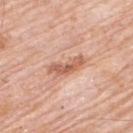This lesion was catalogued during total-body skin photography and was not selected for biopsy.
Longest diameter approximately 4.5 mm.
Cropped from a whole-body photographic skin survey; the tile spans about 15 mm.
A male patient aged approximately 80.
The lesion is located on the upper back.
Captured under white-light illumination.